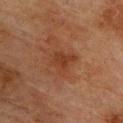Q: What kind of image is this?
A: ~15 mm tile from a whole-body skin photo
Q: What is the anatomic site?
A: the chest
Q: How was the tile lit?
A: cross-polarized illumination
Q: Who is the patient?
A: male, aged around 75